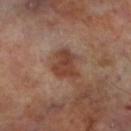The lesion was photographed on a routine skin check and not biopsied; there is no pathology result. A male subject aged approximately 70. A 15 mm close-up tile from a total-body photography series done for melanoma screening. Located on the left lower leg. This is a cross-polarized tile.